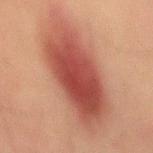Q: Is there a histopathology result?
A: imaged on a skin check; not biopsied
Q: Automated lesion metrics?
A: two-axis asymmetry of about 0.2; roughly 13 lightness units darker than nearby skin and a lesion-to-skin contrast of about 10 (normalized; higher = more distinct); border irregularity of about 3 on a 0–10 scale, internal color variation of about 6 on a 0–10 scale, and a peripheral color-asymmetry measure near 2; a nevus-likeness score of about 100/100 and a detector confidence of about 100 out of 100 that the crop contains a lesion
Q: How was this image acquired?
A: total-body-photography crop, ~15 mm field of view
Q: What lighting was used for the tile?
A: cross-polarized
Q: Where on the body is the lesion?
A: the mid back
Q: What are the patient's age and sex?
A: male, in their mid- to late 40s
Q: Lesion size?
A: ≈11.5 mm